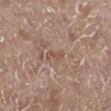Part of a total-body skin-imaging series; this lesion was reviewed on a skin check and was not flagged for biopsy.
A 15 mm close-up extracted from a 3D total-body photography capture.
The tile uses white-light illumination.
Located on the left lower leg.
A male subject, aged 68–72.
Measured at roughly 2.5 mm in maximum diameter.